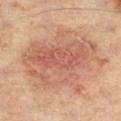biopsy_status: not biopsied; imaged during a skin examination
patient:
  sex: male
  age_approx: 60
site: left thigh
image:
  source: total-body photography crop
  field_of_view_mm: 15
lighting: cross-polarized
lesion_size:
  long_diameter_mm_approx: 9.5